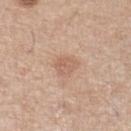<tbp_lesion>
  <biopsy_status>not biopsied; imaged during a skin examination</biopsy_status>
  <automated_metrics>
    <area_mm2_approx>5.5</area_mm2_approx>
    <eccentricity>0.4</eccentricity>
    <shape_asymmetry>0.25</shape_asymmetry>
    <cielab_L>61</cielab_L>
    <cielab_a>20</cielab_a>
    <cielab_b>29</cielab_b>
    <vs_skin_darker_L>8.0</vs_skin_darker_L>
    <border_irregularity_0_10>2.0</border_irregularity_0_10>
    <peripheral_color_asymmetry>1.0</peripheral_color_asymmetry>
    <nevus_likeness_0_100>45</nevus_likeness_0_100>
    <lesion_detection_confidence_0_100>100</lesion_detection_confidence_0_100>
  </automated_metrics>
  <patient>
    <sex>female</sex>
    <age_approx>45</age_approx>
  </patient>
  <lesion_size>
    <long_diameter_mm_approx>3.0</long_diameter_mm_approx>
  </lesion_size>
  <image>
    <source>total-body photography crop</source>
    <field_of_view_mm>15</field_of_view_mm>
  </image>
  <site>leg</site>
</tbp_lesion>